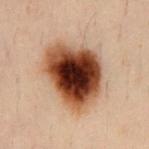Q: Is there a histopathology result?
A: catalogued during a skin exam; not biopsied
Q: What lighting was used for the tile?
A: cross-polarized
Q: What is the anatomic site?
A: the chest
Q: What are the patient's age and sex?
A: male, aged 28–32
Q: What is the imaging modality?
A: ~15 mm tile from a whole-body skin photo
Q: How large is the lesion?
A: ≈8 mm